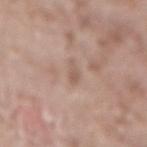Impression: Imaged during a routine full-body skin examination; the lesion was not biopsied and no histopathology is available. Context: The lesion is located on the lower back. A 15 mm close-up tile from a total-body photography series done for melanoma screening. A male subject aged 53 to 57.